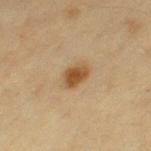Captured during whole-body skin photography for melanoma surveillance; the lesion was not biopsied. The tile uses cross-polarized illumination. A female patient, approximately 55 years of age. About 3 mm across. A 15 mm crop from a total-body photograph taken for skin-cancer surveillance. The lesion is located on the left thigh. Automated image analysis of the tile measured an outline eccentricity of about 0.8 (0 = round, 1 = elongated) and a symmetry-axis asymmetry near 0.25. The software also gave an average lesion color of about L≈44 a*≈17 b*≈34 (CIELAB) and roughly 11 lightness units darker than nearby skin. And it measured border irregularity of about 2 on a 0–10 scale and peripheral color asymmetry of about 1. And it measured a nevus-likeness score of about 100/100 and a lesion-detection confidence of about 100/100.